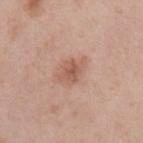Assessment:
Recorded during total-body skin imaging; not selected for excision or biopsy.
Image and clinical context:
On the left upper arm. The lesion's longest dimension is about 3 mm. A female patient, approximately 40 years of age. The tile uses white-light illumination. An algorithmic analysis of the crop reported an outline eccentricity of about 0.6 (0 = round, 1 = elongated) and a shape-asymmetry score of about 0.25 (0 = symmetric). And it measured a lesion–skin lightness drop of about 9 and a normalized border contrast of about 6.5. It also reported lesion-presence confidence of about 100/100. Cropped from a whole-body photographic skin survey; the tile spans about 15 mm.